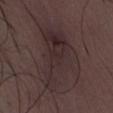Imaged during a routine full-body skin examination; the lesion was not biopsied and no histopathology is available. A 15 mm crop from a total-body photograph taken for skin-cancer surveillance. An algorithmic analysis of the crop reported a lesion color around L≈28 a*≈14 b*≈13 in CIELAB, about 6 CIELAB-L* units darker than the surrounding skin, and a normalized lesion–skin contrast near 6.5. The analysis additionally found a border-irregularity rating of about 6/10, internal color variation of about 4.5 on a 0–10 scale, and peripheral color asymmetry of about 1.5. And it measured an automated nevus-likeness rating near 0 out of 100 and lesion-presence confidence of about 80/100. Captured under white-light illumination. The patient is a male aged around 30. From the front of the torso. Longest diameter approximately 9 mm.